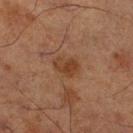Impression: The lesion was tiled from a total-body skin photograph and was not biopsied. Context: A region of skin cropped from a whole-body photographic capture, roughly 15 mm wide. A male patient approximately 70 years of age. Automated image analysis of the tile measured a lesion area of about 5.5 mm², an eccentricity of roughly 0.7, and a shape-asymmetry score of about 0.25 (0 = symmetric). It also reported a mean CIELAB color near L≈32 a*≈18 b*≈27, about 7 CIELAB-L* units darker than the surrounding skin, and a normalized lesion–skin contrast near 7. And it measured border irregularity of about 2.5 on a 0–10 scale, internal color variation of about 3 on a 0–10 scale, and peripheral color asymmetry of about 1. The software also gave a nevus-likeness score of about 35/100 and a detector confidence of about 100 out of 100 that the crop contains a lesion. Imaged with cross-polarized lighting. From the leg.